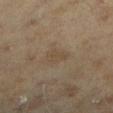The lesion was photographed on a routine skin check and not biopsied; there is no pathology result. A region of skin cropped from a whole-body photographic capture, roughly 15 mm wide. A female patient in their 60s. Located on the left lower leg.